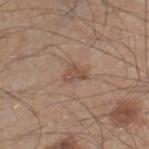{"biopsy_status": "not biopsied; imaged during a skin examination", "lesion_size": {"long_diameter_mm_approx": 2.5}, "lighting": "white-light", "site": "right lower leg", "patient": {"sex": "male", "age_approx": 45}, "image": {"source": "total-body photography crop", "field_of_view_mm": 15}, "automated_metrics": {"area_mm2_approx": 3.5, "eccentricity": 0.7, "shape_asymmetry": 0.45, "cielab_L": 50, "cielab_a": 17, "cielab_b": 27, "vs_skin_darker_L": 8.0, "vs_skin_contrast_norm": 6.0, "border_irregularity_0_10": 4.0, "color_variation_0_10": 2.0, "peripheral_color_asymmetry": 1.0}}